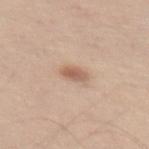This lesion was catalogued during total-body skin photography and was not selected for biopsy.
A male patient roughly 75 years of age.
This is a white-light tile.
Automated image analysis of the tile measured a lesion area of about 4 mm², an outline eccentricity of about 0.8 (0 = round, 1 = elongated), and a shape-asymmetry score of about 0.2 (0 = symmetric). And it measured a lesion-to-skin contrast of about 7.5 (normalized; higher = more distinct). The software also gave an automated nevus-likeness rating near 90 out of 100.
Located on the abdomen.
A roughly 15 mm field-of-view crop from a total-body skin photograph.
About 2.5 mm across.